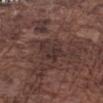notes = imaged on a skin check; not biopsied | site = the left forearm | patient = male, aged 73–77 | image source = 15 mm crop, total-body photography.